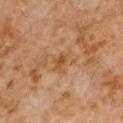Case summary:
- follow-up — total-body-photography surveillance lesion; no biopsy
- location — the right upper arm
- patient — male, about 60 years old
- imaging modality — 15 mm crop, total-body photography